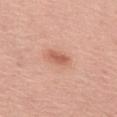* notes — catalogued during a skin exam; not biopsied
* image source — ~15 mm crop, total-body skin-cancer survey
* diameter — ~3 mm (longest diameter)
* subject — female, roughly 65 years of age
* location — the mid back